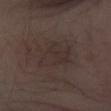Part of a total-body skin-imaging series; this lesion was reviewed on a skin check and was not flagged for biopsy.
The subject is a male aged 48 to 52.
Cropped from a total-body skin-imaging series; the visible field is about 15 mm.